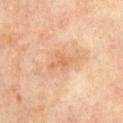Acquisition and patient details:
A male subject, approximately 70 years of age. This image is a 15 mm lesion crop taken from a total-body photograph. The total-body-photography lesion software estimated a lesion color around L≈64 a*≈22 b*≈37 in CIELAB, a lesion–skin lightness drop of about 8, and a normalized border contrast of about 5. It also reported an automated nevus-likeness rating near 0 out of 100 and lesion-presence confidence of about 100/100. The lesion is on the chest. About 2.5 mm across.